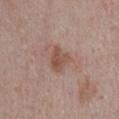Recorded during total-body skin imaging; not selected for excision or biopsy. A 15 mm close-up tile from a total-body photography series done for melanoma screening. The tile uses white-light illumination. The lesion's longest dimension is about 3.5 mm. Automated image analysis of the tile measured an area of roughly 6 mm², an outline eccentricity of about 0.65 (0 = round, 1 = elongated), and a shape-asymmetry score of about 0.35 (0 = symmetric). It also reported border irregularity of about 3.5 on a 0–10 scale, internal color variation of about 3 on a 0–10 scale, and radial color variation of about 1. The analysis additionally found a classifier nevus-likeness of about 0/100 and a detector confidence of about 100 out of 100 that the crop contains a lesion. From the chest. A male subject, approximately 55 years of age.